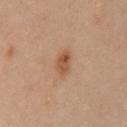The lesion was photographed on a routine skin check and not biopsied; there is no pathology result. A close-up tile cropped from a whole-body skin photograph, about 15 mm across. About 3.5 mm across. Imaged with cross-polarized lighting. The lesion is located on the chest. A male patient, aged approximately 65.